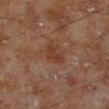{
  "biopsy_status": "not biopsied; imaged during a skin examination",
  "patient": {
    "sex": "male",
    "age_approx": 70
  },
  "image": {
    "source": "total-body photography crop",
    "field_of_view_mm": 15
  },
  "site": "left lower leg",
  "automated_metrics": {
    "nevus_likeness_0_100": 0,
    "lesion_detection_confidence_0_100": 100
  }
}